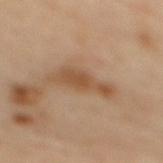Impression:
The lesion was tiled from a total-body skin photograph and was not biopsied.
Acquisition and patient details:
Approximately 5 mm at its widest. A male subject roughly 65 years of age. A close-up tile cropped from a whole-body skin photograph, about 15 mm across. The lesion is located on the mid back.